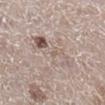A 15 mm crop from a total-body photograph taken for skin-cancer surveillance.
Captured under white-light illumination.
The lesion is on the right lower leg.
A female patient in their 50s.
About 6 mm across.
Automated image analysis of the tile measured a lesion area of about 15 mm². The software also gave a nevus-likeness score of about 5/100 and a detector confidence of about 55 out of 100 that the crop contains a lesion.